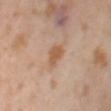| key | value |
|---|---|
| site | the back |
| image source | ~15 mm crop, total-body skin-cancer survey |
| diameter | ~3 mm (longest diameter) |
| subject | female, aged 53 to 57 |
| tile lighting | cross-polarized illumination |